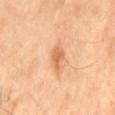Q: What is the anatomic site?
A: the lower back
Q: Patient demographics?
A: male, aged 68 to 72
Q: What is the lesion's diameter?
A: ≈2.5 mm
Q: What lighting was used for the tile?
A: cross-polarized
Q: How was this image acquired?
A: ~15 mm tile from a whole-body skin photo